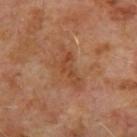Assessment: This lesion was catalogued during total-body skin photography and was not selected for biopsy. Background: The tile uses cross-polarized illumination. The lesion's longest dimension is about 5 mm. The patient is a male aged 63–67. The lesion is on the upper back. Automated image analysis of the tile measured a nevus-likeness score of about 0/100 and a lesion-detection confidence of about 100/100. A 15 mm close-up tile from a total-body photography series done for melanoma screening.